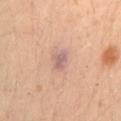The lesion was photographed on a routine skin check and not biopsied; there is no pathology result. A lesion tile, about 15 mm wide, cut from a 3D total-body photograph. Longest diameter approximately 2.5 mm. The total-body-photography lesion software estimated an area of roughly 4.5 mm², a shape eccentricity near 0.7, and two-axis asymmetry of about 0.2. It also reported a lesion-to-skin contrast of about 7 (normalized; higher = more distinct). And it measured border irregularity of about 2 on a 0–10 scale, a color-variation rating of about 2.5/10, and a peripheral color-asymmetry measure near 1. From the lower back. A male patient in their 30s. The tile uses cross-polarized illumination.